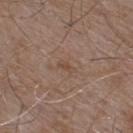{
  "biopsy_status": "not biopsied; imaged during a skin examination",
  "image": {
    "source": "total-body photography crop",
    "field_of_view_mm": 15
  },
  "lesion_size": {
    "long_diameter_mm_approx": 2.5
  },
  "site": "upper back",
  "patient": {
    "sex": "male",
    "age_approx": 55
  },
  "lighting": "white-light"
}